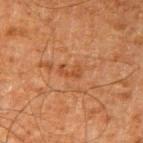Imaged during a routine full-body skin examination; the lesion was not biopsied and no histopathology is available.
A male patient, aged 63–67.
Cropped from a total-body skin-imaging series; the visible field is about 15 mm.
On the right upper arm.